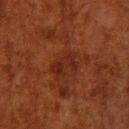Impression:
Part of a total-body skin-imaging series; this lesion was reviewed on a skin check and was not flagged for biopsy.
Background:
Imaged with cross-polarized lighting. A 15 mm crop from a total-body photograph taken for skin-cancer surveillance. An algorithmic analysis of the crop reported an area of roughly 5.5 mm² and a shape-asymmetry score of about 0.3 (0 = symmetric). The analysis additionally found a mean CIELAB color near L≈20 a*≈22 b*≈25 and a lesion-to-skin contrast of about 6.5 (normalized; higher = more distinct). The software also gave a border-irregularity rating of about 4/10, a within-lesion color-variation index near 3/10, and a peripheral color-asymmetry measure near 1. The lesion is located on the right lower leg. The lesion's longest dimension is about 3 mm. The subject is a male roughly 80 years of age.